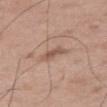Assessment:
Imaged during a routine full-body skin examination; the lesion was not biopsied and no histopathology is available.
Clinical summary:
The lesion's longest dimension is about 3.5 mm. Automated image analysis of the tile measured an area of roughly 4.5 mm² and a shape-asymmetry score of about 0.35 (0 = symmetric). And it measured a classifier nevus-likeness of about 0/100. The patient is a male aged 48–52. This is a white-light tile. From the right thigh. A close-up tile cropped from a whole-body skin photograph, about 15 mm across.